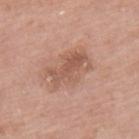Impression: The lesion was tiled from a total-body skin photograph and was not biopsied. Background: A female patient aged approximately 60. The lesion is on the back. Approximately 5.5 mm at its widest. This image is a 15 mm lesion crop taken from a total-body photograph. The total-body-photography lesion software estimated a border-irregularity rating of about 4.5/10, a within-lesion color-variation index near 5/10, and a peripheral color-asymmetry measure near 2. Captured under white-light illumination.